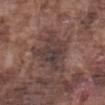No biopsy was performed on this lesion — it was imaged during a full skin examination and was not determined to be concerning.